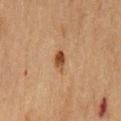notes: no biopsy performed (imaged during a skin exam) | diameter: about 2.5 mm | location: the chest | TBP lesion metrics: a lesion area of about 3 mm², an eccentricity of roughly 0.85, and two-axis asymmetry of about 0.2 | patient: male, aged approximately 75 | imaging modality: ~15 mm crop, total-body skin-cancer survey | illumination: cross-polarized.